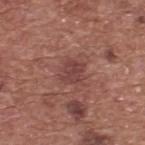Q: Was a biopsy performed?
A: total-body-photography surveillance lesion; no biopsy
Q: What is the lesion's diameter?
A: ≈2.5 mm
Q: What did automated image analysis measure?
A: a mean CIELAB color near L≈42 a*≈24 b*≈23 and a lesion–skin lightness drop of about 8; an automated nevus-likeness rating near 10 out of 100
Q: What kind of image is this?
A: total-body-photography crop, ~15 mm field of view
Q: Who is the patient?
A: male, aged 73–77
Q: What lighting was used for the tile?
A: white-light
Q: Where on the body is the lesion?
A: the upper back